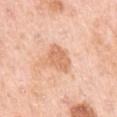Imaged during a routine full-body skin examination; the lesion was not biopsied and no histopathology is available. About 3.5 mm across. The patient is a female aged around 50. A region of skin cropped from a whole-body photographic capture, roughly 15 mm wide. Captured under white-light illumination. The lesion is located on the left upper arm. Automated image analysis of the tile measured a shape-asymmetry score of about 0.25 (0 = symmetric). It also reported a mean CIELAB color near L≈68 a*≈23 b*≈36, about 10 CIELAB-L* units darker than the surrounding skin, and a normalized lesion–skin contrast near 6.5. The analysis additionally found a nevus-likeness score of about 20/100 and lesion-presence confidence of about 100/100.